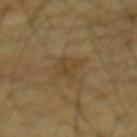Q: Was this lesion biopsied?
A: catalogued during a skin exam; not biopsied
Q: Where on the body is the lesion?
A: the back
Q: How was the tile lit?
A: cross-polarized
Q: What kind of image is this?
A: total-body-photography crop, ~15 mm field of view
Q: How large is the lesion?
A: ~3 mm (longest diameter)
Q: Patient demographics?
A: male, about 65 years old